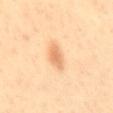Recorded during total-body skin imaging; not selected for excision or biopsy.
From the back.
A female patient aged 38 to 42.
This image is a 15 mm lesion crop taken from a total-body photograph.
An algorithmic analysis of the crop reported an average lesion color of about L≈75 a*≈24 b*≈42 (CIELAB), a lesion–skin lightness drop of about 12, and a lesion-to-skin contrast of about 6.5 (normalized; higher = more distinct). The analysis additionally found internal color variation of about 2 on a 0–10 scale and a peripheral color-asymmetry measure near 0.5.
The lesion's longest dimension is about 3.5 mm.
Captured under cross-polarized illumination.